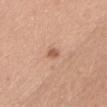Assessment:
Recorded during total-body skin imaging; not selected for excision or biopsy.
Context:
Cropped from a whole-body photographic skin survey; the tile spans about 15 mm. Captured under white-light illumination. Longest diameter approximately 1.5 mm. A female subject, roughly 40 years of age. The total-body-photography lesion software estimated a lesion area of about 2 mm² and an outline eccentricity of about 0.5 (0 = round, 1 = elongated). And it measured a border-irregularity rating of about 2.5/10, a within-lesion color-variation index near 0.5/10, and radial color variation of about 0. Located on the head or neck.